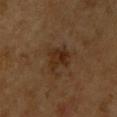{"biopsy_status": "not biopsied; imaged during a skin examination", "site": "right upper arm", "automated_metrics": {"border_irregularity_0_10": 4.0, "color_variation_0_10": 4.5, "peripheral_color_asymmetry": 1.5, "nevus_likeness_0_100": 40, "lesion_detection_confidence_0_100": 100}, "patient": {"sex": "male", "age_approx": 60}, "lighting": "cross-polarized", "image": {"source": "total-body photography crop", "field_of_view_mm": 15}, "lesion_size": {"long_diameter_mm_approx": 3.0}}